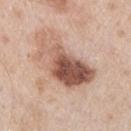Assessment: Imaged during a routine full-body skin examination; the lesion was not biopsied and no histopathology is available. Background: A 15 mm close-up extracted from a 3D total-body photography capture. A male patient, aged 53 to 57. Located on the left upper arm.